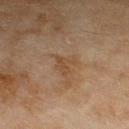Assessment:
This lesion was catalogued during total-body skin photography and was not selected for biopsy.
Acquisition and patient details:
The lesion is on the left lower leg. A lesion tile, about 15 mm wide, cut from a 3D total-body photograph. A female patient aged approximately 60. Captured under cross-polarized illumination. Automated tile analysis of the lesion measured a lesion area of about 4.5 mm², a shape eccentricity near 0.5, and two-axis asymmetry of about 0.45. The software also gave an average lesion color of about L≈43 a*≈16 b*≈30 (CIELAB) and a normalized lesion–skin contrast near 5.5. The software also gave a border-irregularity rating of about 5.5/10, internal color variation of about 1.5 on a 0–10 scale, and radial color variation of about 0.5. It also reported a nevus-likeness score of about 0/100. The recorded lesion diameter is about 3 mm.